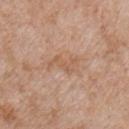A male subject aged around 65. A region of skin cropped from a whole-body photographic capture, roughly 15 mm wide. About 4 mm across. The lesion is on the chest. Captured under white-light illumination.